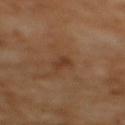| feature | finding |
|---|---|
| biopsy status | imaged on a skin check; not biopsied |
| tile lighting | cross-polarized |
| site | the upper back |
| patient | female, aged 53–57 |
| imaging modality | total-body-photography crop, ~15 mm field of view |
| image-analysis metrics | a footprint of about 2.5 mm² and a symmetry-axis asymmetry near 0.35; a border-irregularity index near 4/10, a color-variation rating of about 0/10, and a peripheral color-asymmetry measure near 0 |
| diameter | about 2.5 mm |